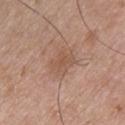biopsy status — total-body-photography surveillance lesion; no biopsy
subject — male, approximately 50 years of age
lighting — white-light illumination
body site — the chest
image — 15 mm crop, total-body photography